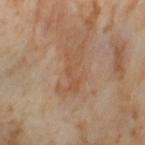{
  "biopsy_status": "not biopsied; imaged during a skin examination",
  "site": "left thigh",
  "automated_metrics": {
    "area_mm2_approx": 6.0,
    "eccentricity": 0.95,
    "shape_asymmetry": 0.5,
    "vs_skin_darker_L": 6.0,
    "vs_skin_contrast_norm": 5.5,
    "nevus_likeness_0_100": 0,
    "lesion_detection_confidence_0_100": 100
  },
  "lighting": "cross-polarized",
  "image": {
    "source": "total-body photography crop",
    "field_of_view_mm": 15
  },
  "patient": {
    "sex": "female",
    "age_approx": 55
  }
}